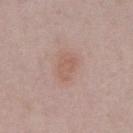Q: Was this lesion biopsied?
A: no biopsy performed (imaged during a skin exam)
Q: What lighting was used for the tile?
A: white-light
Q: Lesion size?
A: ≈3.5 mm
Q: What is the imaging modality?
A: 15 mm crop, total-body photography
Q: What is the anatomic site?
A: the abdomen
Q: Patient demographics?
A: male, about 60 years old